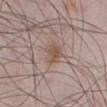Q: Was this lesion biopsied?
A: total-body-photography surveillance lesion; no biopsy
Q: What kind of image is this?
A: 15 mm crop, total-body photography
Q: Lesion size?
A: ≈3 mm
Q: Where on the body is the lesion?
A: the right lower leg
Q: What did automated image analysis measure?
A: a nevus-likeness score of about 65/100 and a lesion-detection confidence of about 100/100
Q: Patient demographics?
A: male, aged 48 to 52